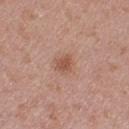Impression: Captured during whole-body skin photography for melanoma surveillance; the lesion was not biopsied. Background: The patient is a female aged 38–42. A close-up tile cropped from a whole-body skin photograph, about 15 mm across. From the left upper arm.